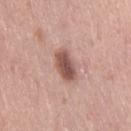The tile uses white-light illumination.
From the right thigh.
A female patient aged 38–42.
An algorithmic analysis of the crop reported a lesion area of about 7.5 mm², an outline eccentricity of about 0.85 (0 = round, 1 = elongated), and a symmetry-axis asymmetry near 0.15. The software also gave internal color variation of about 3.5 on a 0–10 scale and radial color variation of about 1. It also reported an automated nevus-likeness rating near 90 out of 100 and a detector confidence of about 100 out of 100 that the crop contains a lesion.
The recorded lesion diameter is about 4 mm.
A roughly 15 mm field-of-view crop from a total-body skin photograph.